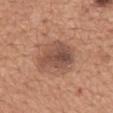follow-up: total-body-photography surveillance lesion; no biopsy | patient: female, aged 53 to 57 | tile lighting: white-light | size: about 5.5 mm | TBP lesion metrics: a border-irregularity rating of about 3/10, internal color variation of about 5.5 on a 0–10 scale, and a peripheral color-asymmetry measure near 2 | anatomic site: the mid back | image: ~15 mm crop, total-body skin-cancer survey.